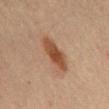biopsy status: imaged on a skin check; not biopsied
anatomic site: the mid back
patient: male, aged 68–72
size: ≈5 mm
illumination: cross-polarized illumination
imaging modality: total-body-photography crop, ~15 mm field of view